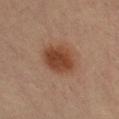Notes:
– notes: total-body-photography surveillance lesion; no biopsy
– anatomic site: the leg
– patient: female, aged 38–42
– image: ~15 mm tile from a whole-body skin photo
– lesion size: ≈4.5 mm
– TBP lesion metrics: a border-irregularity rating of about 1/10 and a peripheral color-asymmetry measure near 1.5; a classifier nevus-likeness of about 100/100 and a detector confidence of about 100 out of 100 that the crop contains a lesion